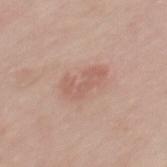Part of a total-body skin-imaging series; this lesion was reviewed on a skin check and was not flagged for biopsy.